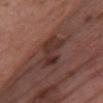Notes:
- illumination — white-light illumination
- lesion diameter — ≈5 mm
- image source — 15 mm crop, total-body photography
- subject — female, aged around 60
- site — the chest